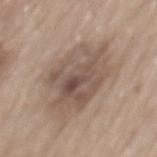Impression: The lesion was photographed on a routine skin check and not biopsied; there is no pathology result. Image and clinical context: A male subject aged approximately 80. This is a white-light tile. A lesion tile, about 15 mm wide, cut from a 3D total-body photograph. The recorded lesion diameter is about 7.5 mm. From the mid back. The lesion-visualizer software estimated a symmetry-axis asymmetry near 0.2. And it measured a mean CIELAB color near L≈52 a*≈14 b*≈24, about 10 CIELAB-L* units darker than the surrounding skin, and a normalized border contrast of about 7.5. The software also gave a color-variation rating of about 7/10. The analysis additionally found a lesion-detection confidence of about 100/100.